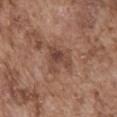notes: imaged on a skin check; not biopsied
imaging modality: 15 mm crop, total-body photography
body site: the abdomen
subject: male, aged 73–77